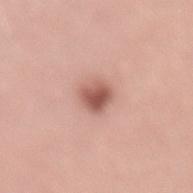Acquisition and patient details: A 15 mm close-up extracted from a 3D total-body photography capture. The recorded lesion diameter is about 2.5 mm. The lesion is on the lower back. A male patient aged 43–47. An algorithmic analysis of the crop reported an area of roughly 5.5 mm², a shape eccentricity near 0.35, and a symmetry-axis asymmetry near 0.2. The software also gave border irregularity of about 1.5 on a 0–10 scale and a within-lesion color-variation index near 5/10. The analysis additionally found a nevus-likeness score of about 95/100 and a detector confidence of about 100 out of 100 that the crop contains a lesion.